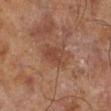The lesion was photographed on a routine skin check and not biopsied; there is no pathology result.